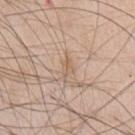Case summary:
– biopsy status: imaged on a skin check; not biopsied
– image: 15 mm crop, total-body photography
– patient: male, approximately 60 years of age
– site: the chest
– size: ~2.5 mm (longest diameter)